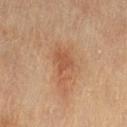Recorded during total-body skin imaging; not selected for excision or biopsy.
About 4 mm across.
A 15 mm close-up extracted from a 3D total-body photography capture.
The lesion-visualizer software estimated an area of roughly 7.5 mm² and a shape-asymmetry score of about 0.45 (0 = symmetric). The analysis additionally found an automated nevus-likeness rating near 20 out of 100 and lesion-presence confidence of about 100/100.
Imaged with cross-polarized lighting.
On the left thigh.
A female patient approximately 55 years of age.